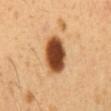No biopsy was performed on this lesion — it was imaged during a full skin examination and was not determined to be concerning. Cropped from a total-body skin-imaging series; the visible field is about 15 mm. Approximately 5 mm at its widest. Located on the abdomen. An algorithmic analysis of the crop reported an outline eccentricity of about 0.8 (0 = round, 1 = elongated) and a symmetry-axis asymmetry near 0.1. And it measured about 24 CIELAB-L* units darker than the surrounding skin and a normalized border contrast of about 15.5. And it measured a border-irregularity rating of about 1/10, internal color variation of about 6.5 on a 0–10 scale, and peripheral color asymmetry of about 1.5. And it measured an automated nevus-likeness rating near 100 out of 100 and a detector confidence of about 100 out of 100 that the crop contains a lesion. A male subject, approximately 50 years of age. Captured under cross-polarized illumination.